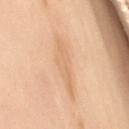| field | value |
|---|---|
| notes | no biopsy performed (imaged during a skin exam) |
| TBP lesion metrics | an average lesion color of about L≈60 a*≈17 b*≈32 (CIELAB), about 6 CIELAB-L* units darker than the surrounding skin, and a normalized border contrast of about 4.5; a color-variation rating of about 1.5/10 and radial color variation of about 0.5 |
| lesion diameter | ~6 mm (longest diameter) |
| patient | female, aged 58 to 62 |
| acquisition | ~15 mm tile from a whole-body skin photo |
| site | the lower back |